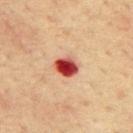Q: Was this lesion biopsied?
A: no biopsy performed (imaged during a skin exam)
Q: What are the patient's age and sex?
A: male, aged 63–67
Q: How was the tile lit?
A: cross-polarized illumination
Q: What is the imaging modality?
A: ~15 mm crop, total-body skin-cancer survey
Q: Lesion location?
A: the mid back
Q: What is the lesion's diameter?
A: about 3 mm